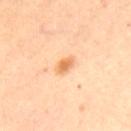image source: 15 mm crop, total-body photography
anatomic site: the upper back
lesion size: ~2.5 mm (longest diameter)
image-analysis metrics: a footprint of about 3.5 mm², a shape eccentricity near 0.8, and two-axis asymmetry of about 0.25; an average lesion color of about L≈71 a*≈25 b*≈44 (CIELAB), about 12 CIELAB-L* units darker than the surrounding skin, and a normalized lesion–skin contrast near 8; border irregularity of about 2 on a 0–10 scale, a within-lesion color-variation index near 3/10, and a peripheral color-asymmetry measure near 1; a classifier nevus-likeness of about 80/100 and a detector confidence of about 100 out of 100 that the crop contains a lesion
lighting: cross-polarized illumination
patient: female, aged 43 to 47